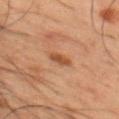Clinical impression:
Recorded during total-body skin imaging; not selected for excision or biopsy.
Acquisition and patient details:
A male subject, aged 48 to 52. About 2.5 mm across. On the front of the torso. This is a cross-polarized tile. Automated image analysis of the tile measured a footprint of about 3 mm² and a symmetry-axis asymmetry near 0.25. It also reported an average lesion color of about L≈37 a*≈19 b*≈28 (CIELAB), roughly 8 lightness units darker than nearby skin, and a normalized border contrast of about 8. The analysis additionally found a nevus-likeness score of about 55/100 and a detector confidence of about 100 out of 100 that the crop contains a lesion. Cropped from a whole-body photographic skin survey; the tile spans about 15 mm.